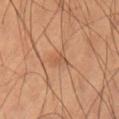No biopsy was performed on this lesion — it was imaged during a full skin examination and was not determined to be concerning. A male subject, in their 60s. Located on the lower back. A 15 mm close-up tile from a total-body photography series done for melanoma screening.